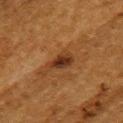biopsy status: no biopsy performed (imaged during a skin exam); image source: ~15 mm crop, total-body skin-cancer survey; body site: the back; patient: female, roughly 50 years of age; lesion size: ~4 mm (longest diameter); illumination: cross-polarized illumination.